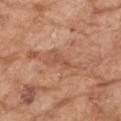The lesion was tiled from a total-body skin photograph and was not biopsied. A female patient, aged around 75. Cropped from a total-body skin-imaging series; the visible field is about 15 mm. Automated image analysis of the tile measured a lesion area of about 3 mm², an eccentricity of roughly 0.9, and a shape-asymmetry score of about 0.55 (0 = symmetric). And it measured an automated nevus-likeness rating near 0 out of 100 and a lesion-detection confidence of about 100/100. Located on the right forearm. Longest diameter approximately 3.5 mm.